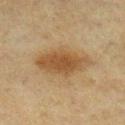Captured during whole-body skin photography for melanoma surveillance; the lesion was not biopsied.
The recorded lesion diameter is about 6.5 mm.
Automated tile analysis of the lesion measured a lesion color around L≈43 a*≈15 b*≈32 in CIELAB and roughly 10 lightness units darker than nearby skin. The software also gave a border-irregularity index near 2.5/10, internal color variation of about 3 on a 0–10 scale, and peripheral color asymmetry of about 1.
The patient is a female about 55 years old.
A close-up tile cropped from a whole-body skin photograph, about 15 mm across.
Located on the right lower leg.
This is a cross-polarized tile.